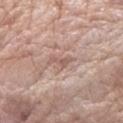Clinical impression:
Part of a total-body skin-imaging series; this lesion was reviewed on a skin check and was not flagged for biopsy.
Image and clinical context:
The lesion's longest dimension is about 3 mm. This image is a 15 mm lesion crop taken from a total-body photograph. A female patient aged approximately 70. The lesion is located on the left forearm.